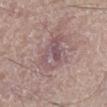biopsy status — catalogued during a skin exam; not biopsied | patient — male, aged 53–57 | image — ~15 mm crop, total-body skin-cancer survey | body site — the right lower leg.